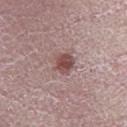  biopsy_status: not biopsied; imaged during a skin examination
  image:
    source: total-body photography crop
    field_of_view_mm: 15
  lighting: white-light
  lesion_size:
    long_diameter_mm_approx: 2.5
  patient:
    sex: male
    age_approx: 20
  site: leg